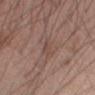Recorded during total-body skin imaging; not selected for excision or biopsy. A close-up tile cropped from a whole-body skin photograph, about 15 mm across. The lesion is located on the left forearm. A male patient about 45 years old.